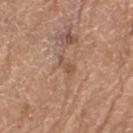Findings:
- biopsy status — no biopsy performed (imaged during a skin exam)